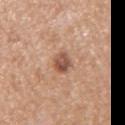This lesion was catalogued during total-body skin photography and was not selected for biopsy.
A region of skin cropped from a whole-body photographic capture, roughly 15 mm wide.
Longest diameter approximately 3 mm.
A female patient approximately 50 years of age.
An algorithmic analysis of the crop reported an automated nevus-likeness rating near 45 out of 100.
Captured under white-light illumination.
The lesion is located on the right upper arm.